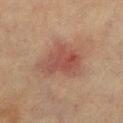No biopsy was performed on this lesion — it was imaged during a full skin examination and was not determined to be concerning.
The tile uses cross-polarized illumination.
A roughly 15 mm field-of-view crop from a total-body skin photograph.
The lesion is on the left lower leg.
A female subject approximately 70 years of age.
An algorithmic analysis of the crop reported a lesion area of about 16 mm². The analysis additionally found an average lesion color of about L≈43 a*≈21 b*≈24 (CIELAB), about 8 CIELAB-L* units darker than the surrounding skin, and a lesion-to-skin contrast of about 7 (normalized; higher = more distinct). The software also gave a border-irregularity rating of about 4/10 and a within-lesion color-variation index near 5/10. And it measured a classifier nevus-likeness of about 5/100.